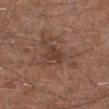No biopsy was performed on this lesion — it was imaged during a full skin examination and was not determined to be concerning.
A male patient approximately 65 years of age.
Approximately 2.5 mm at its widest.
This image is a 15 mm lesion crop taken from a total-body photograph.
Located on the right lower leg.